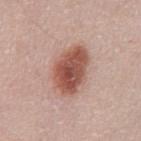Context: Captured under white-light illumination. Longest diameter approximately 6 mm. The patient is a male in their 50s. On the abdomen. The lesion-visualizer software estimated a border-irregularity index near 2/10, a within-lesion color-variation index near 5.5/10, and radial color variation of about 2. A region of skin cropped from a whole-body photographic capture, roughly 15 mm wide.